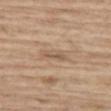Q: Was this lesion biopsied?
A: imaged on a skin check; not biopsied
Q: Where on the body is the lesion?
A: the leg
Q: How large is the lesion?
A: ≈2.5 mm
Q: What kind of image is this?
A: ~15 mm tile from a whole-body skin photo
Q: Automated lesion metrics?
A: a lesion color around L≈56 a*≈16 b*≈30 in CIELAB, a lesion–skin lightness drop of about 8, and a normalized border contrast of about 6; a border-irregularity index near 3.5/10 and a within-lesion color-variation index near 0/10; a classifier nevus-likeness of about 0/100 and a lesion-detection confidence of about 75/100
Q: Patient demographics?
A: male, roughly 70 years of age